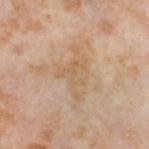Context:
About 6.5 mm across. On the left thigh. An algorithmic analysis of the crop reported a lesion area of about 12 mm² and a shape eccentricity near 0.8. Captured under cross-polarized illumination. A 15 mm close-up tile from a total-body photography series done for melanoma screening. The subject is a female aged 53–57.